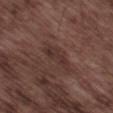notes: catalogued during a skin exam; not biopsied
imaging modality: ~15 mm tile from a whole-body skin photo
automated metrics: a footprint of about 5 mm², a shape eccentricity near 0.95, and two-axis asymmetry of about 0.35; an average lesion color of about L≈32 a*≈18 b*≈20 (CIELAB) and a normalized lesion–skin contrast near 6
lesion diameter: ≈4.5 mm
location: the left thigh
patient: male, aged 73–77